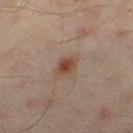Clinical impression: The lesion was tiled from a total-body skin photograph and was not biopsied. Acquisition and patient details: Cropped from a total-body skin-imaging series; the visible field is about 15 mm. Approximately 3 mm at its widest. The patient is a male roughly 45 years of age. Located on the right thigh. Captured under cross-polarized illumination.